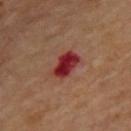imaging modality = ~15 mm tile from a whole-body skin photo | subject = male, in their 70s | location = the upper back.